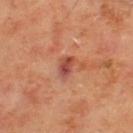Assessment:
The lesion was tiled from a total-body skin photograph and was not biopsied.
Background:
The patient is a male about 65 years old. Located on the upper back. A region of skin cropped from a whole-body photographic capture, roughly 15 mm wide. The lesion's longest dimension is about 2.5 mm. This is a cross-polarized tile.